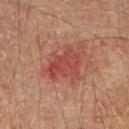workup = imaged on a skin check; not biopsied | patient = male, aged 43 to 47 | image source = ~15 mm crop, total-body skin-cancer survey | anatomic site = the right upper arm.